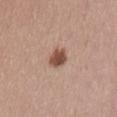workup: no biopsy performed (imaged during a skin exam); image source: total-body-photography crop, ~15 mm field of view; lighting: white-light; subject: female, roughly 40 years of age; anatomic site: the lower back; lesion size: about 2.5 mm.